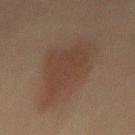Case summary:
* workup · catalogued during a skin exam; not biopsied
* image source · 15 mm crop, total-body photography
* lesion diameter · ~9.5 mm (longest diameter)
* patient · male, aged 58–62
* tile lighting · cross-polarized
* automated metrics · a lesion area of about 31 mm² and an eccentricity of roughly 0.85; an average lesion color of about L≈33 a*≈14 b*≈21 (CIELAB), roughly 6 lightness units darker than nearby skin, and a normalized border contrast of about 6; internal color variation of about 2.5 on a 0–10 scale and a peripheral color-asymmetry measure near 1; a classifier nevus-likeness of about 95/100 and a detector confidence of about 100 out of 100 that the crop contains a lesion
* location · the mid back